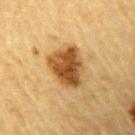biopsy status: total-body-photography surveillance lesion; no biopsy
automated metrics: a mean CIELAB color near L≈45 a*≈19 b*≈38, a lesion–skin lightness drop of about 15, and a normalized border contrast of about 11; internal color variation of about 6.5 on a 0–10 scale and a peripheral color-asymmetry measure near 2; a nevus-likeness score of about 95/100 and a lesion-detection confidence of about 100/100
site: the arm
subject: male, aged around 85 or older
image source: total-body-photography crop, ~15 mm field of view
tile lighting: cross-polarized illumination
lesion diameter: ≈5 mm